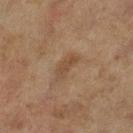follow-up: total-body-photography surveillance lesion; no biopsy | image: ~15 mm tile from a whole-body skin photo | patient: female, approximately 60 years of age | body site: the left lower leg.